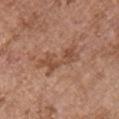Imaged during a routine full-body skin examination; the lesion was not biopsied and no histopathology is available.
A region of skin cropped from a whole-body photographic capture, roughly 15 mm wide.
Located on the right upper arm.
A female patient in their mid- to late 60s.